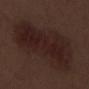– notes: no biopsy performed (imaged during a skin exam)
– lighting: white-light
– subject: male, aged 68–72
– diameter: ~11.5 mm (longest diameter)
– body site: the right lower leg
– image: 15 mm crop, total-body photography